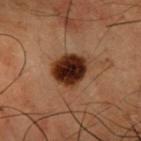No biopsy was performed on this lesion — it was imaged during a full skin examination and was not determined to be concerning. On the front of the torso. Measured at roughly 4 mm in maximum diameter. A male patient, about 50 years old. Imaged with cross-polarized lighting. An algorithmic analysis of the crop reported a lesion color around L≈22 a*≈18 b*≈22 in CIELAB, about 17 CIELAB-L* units darker than the surrounding skin, and a normalized border contrast of about 17. A lesion tile, about 15 mm wide, cut from a 3D total-body photograph.